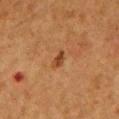Part of a total-body skin-imaging series; this lesion was reviewed on a skin check and was not flagged for biopsy.